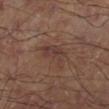Impression:
Captured during whole-body skin photography for melanoma surveillance; the lesion was not biopsied.
Image and clinical context:
Automated tile analysis of the lesion measured border irregularity of about 5.5 on a 0–10 scale, a within-lesion color-variation index near 2.5/10, and peripheral color asymmetry of about 1. And it measured a classifier nevus-likeness of about 15/100 and a detector confidence of about 100 out of 100 that the crop contains a lesion. Located on the left lower leg. A 15 mm crop from a total-body photograph taken for skin-cancer surveillance. About 4.5 mm across. Captured under cross-polarized illumination.